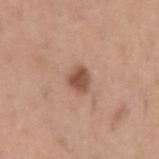follow-up: total-body-photography surveillance lesion; no biopsy
image: ~15 mm crop, total-body skin-cancer survey
anatomic site: the right upper arm
lesion diameter: ≈2.5 mm
patient: male, roughly 60 years of age
automated metrics: a shape-asymmetry score of about 0.2 (0 = symmetric); a border-irregularity index near 1.5/10, a color-variation rating of about 2.5/10, and a peripheral color-asymmetry measure near 1; a nevus-likeness score of about 85/100 and lesion-presence confidence of about 100/100